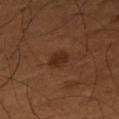Q: Was this lesion biopsied?
A: catalogued during a skin exam; not biopsied
Q: What is the imaging modality?
A: total-body-photography crop, ~15 mm field of view
Q: Who is the patient?
A: male, about 50 years old
Q: Where on the body is the lesion?
A: the right thigh